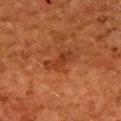lighting: cross-polarized illumination
imaging modality: total-body-photography crop, ~15 mm field of view
size: ≈4 mm
body site: the upper back
patient: female, aged 48–52